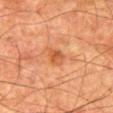The lesion was photographed on a routine skin check and not biopsied; there is no pathology result. This is a cross-polarized tile. The patient is a male aged 78 to 82. Located on the right thigh. Longest diameter approximately 2.5 mm. Cropped from a whole-body photographic skin survey; the tile spans about 15 mm.